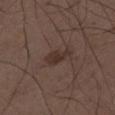  automated_metrics:
    nevus_likeness_0_100: 15
  lighting: white-light
  site: left thigh
  lesion_size:
    long_diameter_mm_approx: 3.5
  image:
    source: total-body photography crop
    field_of_view_mm: 15
  patient:
    sex: male
    age_approx: 50